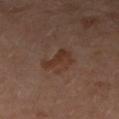workup: imaged on a skin check; not biopsied | lesion diameter: ~3.5 mm (longest diameter) | imaging modality: total-body-photography crop, ~15 mm field of view | anatomic site: the left forearm | subject: female, about 60 years old | illumination: cross-polarized.